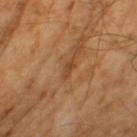Case summary:
– biopsy status: imaged on a skin check; not biopsied
– image source: ~15 mm crop, total-body skin-cancer survey
– subject: male, aged 63 to 67
– illumination: cross-polarized illumination
– image-analysis metrics: a border-irregularity rating of about 3.5/10 and radial color variation of about 0
– lesion diameter: ~2.5 mm (longest diameter)